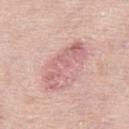No biopsy was performed on this lesion — it was imaged during a full skin examination and was not determined to be concerning.
The patient is a female aged 63 to 67.
The total-body-photography lesion software estimated a normalized lesion–skin contrast near 6.
Longest diameter approximately 6.5 mm.
A 15 mm close-up tile from a total-body photography series done for melanoma screening.
This is a white-light tile.
On the leg.